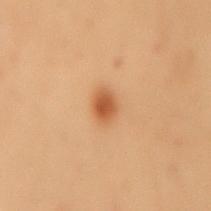Part of a total-body skin-imaging series; this lesion was reviewed on a skin check and was not flagged for biopsy. A female subject about 50 years old. A close-up tile cropped from a whole-body skin photograph, about 15 mm across. About 2.5 mm across. Captured under cross-polarized illumination. An algorithmic analysis of the crop reported a mean CIELAB color near L≈49 a*≈22 b*≈36 and a lesion–skin lightness drop of about 11. On the mid back.